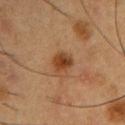This lesion was catalogued during total-body skin photography and was not selected for biopsy. The tile uses cross-polarized illumination. Measured at roughly 2.5 mm in maximum diameter. From the right upper arm. A male patient, aged approximately 55. A 15 mm close-up extracted from a 3D total-body photography capture.